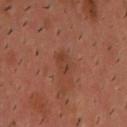Notes:
* follow-up · no biopsy performed (imaged during a skin exam)
* patient · male, about 40 years old
* location · the head or neck
* diameter · about 2.5 mm
* imaging modality · ~15 mm crop, total-body skin-cancer survey
* lighting · cross-polarized illumination
* automated lesion analysis · a shape eccentricity near 0.85 and two-axis asymmetry of about 0.2; a mean CIELAB color near L≈39 a*≈23 b*≈30; a border-irregularity rating of about 2.5/10 and a within-lesion color-variation index near 3/10; a classifier nevus-likeness of about 0/100